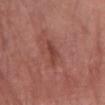biopsy status = no biopsy performed (imaged during a skin exam) | image = 15 mm crop, total-body photography | illumination = white-light | patient = female, in their 70s | site = the left forearm | automated metrics = a lesion area of about 3.5 mm² and an eccentricity of roughly 0.95; border irregularity of about 4.5 on a 0–10 scale, internal color variation of about 1 on a 0–10 scale, and peripheral color asymmetry of about 0.